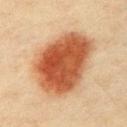This lesion was catalogued during total-body skin photography and was not selected for biopsy. The tile uses cross-polarized illumination. A male subject roughly 40 years of age. About 8.5 mm across. From the chest. A region of skin cropped from a whole-body photographic capture, roughly 15 mm wide.